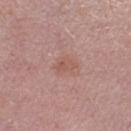Impression:
No biopsy was performed on this lesion — it was imaged during a full skin examination and was not determined to be concerning.
Acquisition and patient details:
On the leg. A roughly 15 mm field-of-view crop from a total-body skin photograph. Measured at roughly 2.5 mm in maximum diameter. The tile uses white-light illumination. A male patient, aged 78–82.